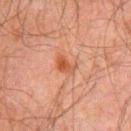Impression: The lesion was tiled from a total-body skin photograph and was not biopsied. Image and clinical context: A 15 mm close-up extracted from a 3D total-body photography capture. This is a cross-polarized tile. Measured at roughly 3 mm in maximum diameter. Located on the front of the torso. The lesion-visualizer software estimated a lesion color around L≈42 a*≈23 b*≈31 in CIELAB and a lesion–skin lightness drop of about 8. It also reported a within-lesion color-variation index near 3/10 and radial color variation of about 1. A male patient, approximately 60 years of age.